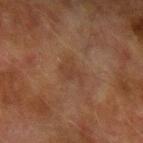Clinical impression: The lesion was photographed on a routine skin check and not biopsied; there is no pathology result. Context: Imaged with cross-polarized lighting. A male subject roughly 75 years of age. The lesion-visualizer software estimated an eccentricity of roughly 0.75. And it measured a border-irregularity index near 5/10, a color-variation rating of about 1.5/10, and peripheral color asymmetry of about 0.5. A 15 mm crop from a total-body photograph taken for skin-cancer surveillance. Approximately 3 mm at its widest. The lesion is on the left upper arm.